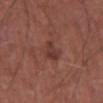Impression: Part of a total-body skin-imaging series; this lesion was reviewed on a skin check and was not flagged for biopsy. Clinical summary: A male subject, in their mid- to late 60s. The tile uses white-light illumination. Automated tile analysis of the lesion measured a lesion color around L≈36 a*≈23 b*≈23 in CIELAB. And it measured a border-irregularity rating of about 3/10, a color-variation rating of about 2.5/10, and a peripheral color-asymmetry measure near 1. It also reported a classifier nevus-likeness of about 5/100. The lesion is located on the abdomen. This image is a 15 mm lesion crop taken from a total-body photograph. Measured at roughly 2.5 mm in maximum diameter.